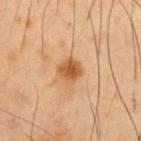follow-up = imaged on a skin check; not biopsied
patient = male, approximately 55 years of age
lighting = cross-polarized illumination
location = the chest
size = ~2.5 mm (longest diameter)
acquisition = 15 mm crop, total-body photography
image-analysis metrics = a border-irregularity rating of about 1.5/10, internal color variation of about 2.5 on a 0–10 scale, and peripheral color asymmetry of about 0.5